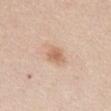Impression:
The lesion was tiled from a total-body skin photograph and was not biopsied.
Image and clinical context:
A region of skin cropped from a whole-body photographic capture, roughly 15 mm wide. Automated tile analysis of the lesion measured a lesion area of about 4.5 mm², an outline eccentricity of about 0.55 (0 = round, 1 = elongated), and a shape-asymmetry score of about 0.25 (0 = symmetric). The software also gave a mean CIELAB color near L≈64 a*≈19 b*≈32 and a lesion-to-skin contrast of about 6.5 (normalized; higher = more distinct). And it measured border irregularity of about 2 on a 0–10 scale, internal color variation of about 2 on a 0–10 scale, and a peripheral color-asymmetry measure near 0.5. The analysis additionally found a nevus-likeness score of about 85/100 and a detector confidence of about 100 out of 100 that the crop contains a lesion. A female patient, aged 43 to 47. The lesion is on the front of the torso. This is a white-light tile.